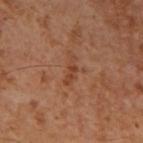Impression:
The lesion was photographed on a routine skin check and not biopsied; there is no pathology result.
Context:
Automated tile analysis of the lesion measured an average lesion color of about L≈43 a*≈24 b*≈33 (CIELAB). The analysis additionally found a border-irregularity index near 5.5/10, internal color variation of about 0 on a 0–10 scale, and radial color variation of about 0. This is a cross-polarized tile. A male subject about 55 years old. A lesion tile, about 15 mm wide, cut from a 3D total-body photograph. The recorded lesion diameter is about 3.5 mm. On the right upper arm.